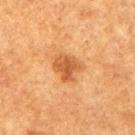Impression: Captured during whole-body skin photography for melanoma surveillance; the lesion was not biopsied. Acquisition and patient details: From the right upper arm. A roughly 15 mm field-of-view crop from a total-body skin photograph. The lesion-visualizer software estimated border irregularity of about 3 on a 0–10 scale and radial color variation of about 1. A male patient, approximately 75 years of age.